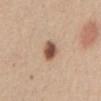The lesion was tiled from a total-body skin photograph and was not biopsied. An algorithmic analysis of the crop reported an eccentricity of roughly 0.65 and a symmetry-axis asymmetry near 0.15. The analysis additionally found a mean CIELAB color near L≈53 a*≈19 b*≈29 and a normalized lesion–skin contrast near 11. And it measured an automated nevus-likeness rating near 100 out of 100 and a lesion-detection confidence of about 100/100. The patient is a female approximately 50 years of age. A 15 mm close-up extracted from a 3D total-body photography capture. The tile uses white-light illumination. On the abdomen. Approximately 3 mm at its widest.